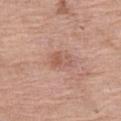Clinical impression: Imaged during a routine full-body skin examination; the lesion was not biopsied and no histopathology is available. Acquisition and patient details: A 15 mm close-up extracted from a 3D total-body photography capture. The lesion is located on the left thigh. The lesion's longest dimension is about 2.5 mm. A female patient in their mid-60s.